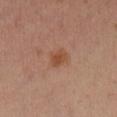Assessment:
Part of a total-body skin-imaging series; this lesion was reviewed on a skin check and was not flagged for biopsy.
Acquisition and patient details:
Measured at roughly 2.5 mm in maximum diameter. The tile uses cross-polarized illumination. The subject is a female approximately 40 years of age. From the left leg. This image is a 15 mm lesion crop taken from a total-body photograph.